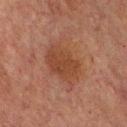| key | value |
|---|---|
| notes | catalogued during a skin exam; not biopsied |
| image source | total-body-photography crop, ~15 mm field of view |
| anatomic site | the upper back |
| tile lighting | cross-polarized |
| subject | male, approximately 65 years of age |
| size | about 5.5 mm |